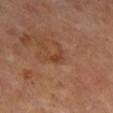Recorded during total-body skin imaging; not selected for excision or biopsy.
The lesion is located on the leg.
A male subject about 65 years old.
The lesion's longest dimension is about 2.5 mm.
A roughly 15 mm field-of-view crop from a total-body skin photograph.